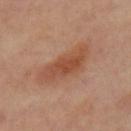| feature | finding |
|---|---|
| workup | imaged on a skin check; not biopsied |
| patient | female, roughly 45 years of age |
| image-analysis metrics | an eccentricity of roughly 0.9 and a symmetry-axis asymmetry near 0.35; lesion-presence confidence of about 100/100 |
| tile lighting | cross-polarized illumination |
| lesion size | about 6.5 mm |
| body site | the leg |
| acquisition | 15 mm crop, total-body photography |